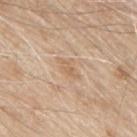The lesion was tiled from a total-body skin photograph and was not biopsied.
Located on the upper back.
A lesion tile, about 15 mm wide, cut from a 3D total-body photograph.
The patient is a male aged 78–82.
The lesion-visualizer software estimated a lesion area of about 3.5 mm² and a symmetry-axis asymmetry near 0.3. The analysis additionally found an average lesion color of about L≈63 a*≈17 b*≈33 (CIELAB) and a normalized border contrast of about 5. It also reported border irregularity of about 3.5 on a 0–10 scale and a within-lesion color-variation index near 2/10.